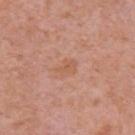Impression: Recorded during total-body skin imaging; not selected for excision or biopsy. Image and clinical context: From the arm. The total-body-photography lesion software estimated an average lesion color of about L≈58 a*≈23 b*≈33 (CIELAB) and roughly 6 lightness units darker than nearby skin. The software also gave a within-lesion color-variation index near 1/10 and radial color variation of about 0.5. The recorded lesion diameter is about 2.5 mm. Imaged with white-light lighting. A female subject approximately 40 years of age. A lesion tile, about 15 mm wide, cut from a 3D total-body photograph.